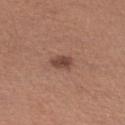| field | value |
|---|---|
| location | the left upper arm |
| subject | female, aged around 30 |
| tile lighting | white-light |
| image source | 15 mm crop, total-body photography |